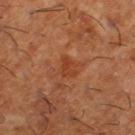Assessment:
No biopsy was performed on this lesion — it was imaged during a full skin examination and was not determined to be concerning.
Acquisition and patient details:
A male patient aged approximately 65. The lesion-visualizer software estimated a lesion color around L≈42 a*≈29 b*≈37 in CIELAB and a lesion-to-skin contrast of about 6 (normalized; higher = more distinct). The lesion is located on the right lower leg. The lesion's longest dimension is about 2.5 mm. A 15 mm close-up extracted from a 3D total-body photography capture. Captured under cross-polarized illumination.